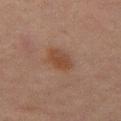The lesion was photographed on a routine skin check and not biopsied; there is no pathology result. The lesion is on the front of the torso. A 15 mm crop from a total-body photograph taken for skin-cancer surveillance. A male subject, in their mid-60s.